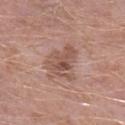* biopsy status — imaged on a skin check; not biopsied
* patient — male, roughly 50 years of age
* body site — the right lower leg
* imaging modality — 15 mm crop, total-body photography